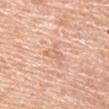<tbp_lesion>
  <biopsy_status>not biopsied; imaged during a skin examination</biopsy_status>
  <image>
    <source>total-body photography crop</source>
    <field_of_view_mm>15</field_of_view_mm>
  </image>
  <lesion_size>
    <long_diameter_mm_approx>3.0</long_diameter_mm_approx>
  </lesion_size>
  <lighting>white-light</lighting>
  <automated_metrics>
    <area_mm2_approx>3.0</area_mm2_approx>
    <eccentricity>0.9</eccentricity>
    <shape_asymmetry>0.35</shape_asymmetry>
    <cielab_L>67</cielab_L>
    <cielab_a>21</cielab_a>
    <cielab_b>34</cielab_b>
    <vs_skin_darker_L>7.0</vs_skin_darker_L>
    <vs_skin_contrast_norm>4.5</vs_skin_contrast_norm>
  </automated_metrics>
  <site>left upper arm</site>
  <patient>
    <sex>male</sex>
    <age_approx>70</age_approx>
  </patient>
</tbp_lesion>